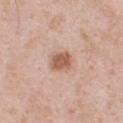<case>
  <biopsy_status>not biopsied; imaged during a skin examination</biopsy_status>
  <patient>
    <sex>male</sex>
    <age_approx>50</age_approx>
  </patient>
  <image>
    <source>total-body photography crop</source>
    <field_of_view_mm>15</field_of_view_mm>
  </image>
  <lesion_size>
    <long_diameter_mm_approx>2.5</long_diameter_mm_approx>
  </lesion_size>
  <site>chest</site>
  <automated_metrics>
    <border_irregularity_0_10>1.5</border_irregularity_0_10>
    <color_variation_0_10>2.0</color_variation_0_10>
    <nevus_likeness_0_100>95</nevus_likeness_0_100>
    <lesion_detection_confidence_0_100>100</lesion_detection_confidence_0_100>
  </automated_metrics>
</case>